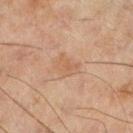notes — imaged on a skin check; not biopsied
patient — male, roughly 45 years of age
image source — ~15 mm tile from a whole-body skin photo
anatomic site — the leg